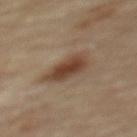site: back
image:
  source: total-body photography crop
  field_of_view_mm: 15
lighting: cross-polarized
patient:
  sex: male
  age_approx: 85+